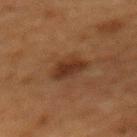workup = imaged on a skin check; not biopsied